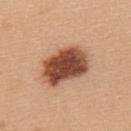notes — total-body-photography surveillance lesion; no biopsy | tile lighting — white-light illumination | acquisition — ~15 mm tile from a whole-body skin photo | anatomic site — the upper back | lesion diameter — ≈6 mm | patient — female, aged 43 to 47.